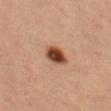Assessment:
The lesion was photographed on a routine skin check and not biopsied; there is no pathology result.
Background:
A 15 mm crop from a total-body photograph taken for skin-cancer surveillance. The subject is a female aged 38–42. The lesion is located on the left thigh. This is a cross-polarized tile.